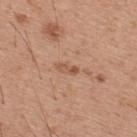A 15 mm close-up extracted from a 3D total-body photography capture. The lesion's longest dimension is about 2.5 mm. From the upper back. An algorithmic analysis of the crop reported a lesion color around L≈54 a*≈22 b*≈33 in CIELAB and a lesion–skin lightness drop of about 9. The software also gave a border-irregularity index near 5.5/10. And it measured a nevus-likeness score of about 0/100 and lesion-presence confidence of about 100/100. The subject is a male in their 30s.